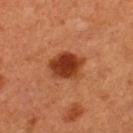Assessment: No biopsy was performed on this lesion — it was imaged during a full skin examination and was not determined to be concerning. Background: A lesion tile, about 15 mm wide, cut from a 3D total-body photograph. A male subject approximately 50 years of age. Captured under cross-polarized illumination. The lesion is on the left thigh. About 4 mm across.